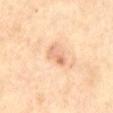follow-up = no biopsy performed (imaged during a skin exam); subject = male, aged approximately 70; location = the abdomen; image = ~15 mm crop, total-body skin-cancer survey.